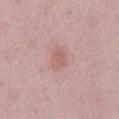Imaged during a routine full-body skin examination; the lesion was not biopsied and no histopathology is available.
Measured at roughly 3.5 mm in maximum diameter.
The lesion is on the abdomen.
The subject is a male approximately 50 years of age.
Cropped from a total-body skin-imaging series; the visible field is about 15 mm.
Imaged with white-light lighting.